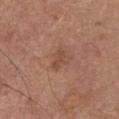This lesion was catalogued during total-body skin photography and was not selected for biopsy. A 15 mm crop from a total-body photograph taken for skin-cancer surveillance. The lesion is on the front of the torso. Automated image analysis of the tile measured a lesion color around L≈47 a*≈22 b*≈29 in CIELAB, about 7 CIELAB-L* units darker than the surrounding skin, and a normalized lesion–skin contrast near 6. A male subject aged 73–77. The tile uses white-light illumination. The lesion's longest dimension is about 2.5 mm.